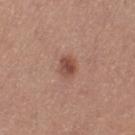follow-up: catalogued during a skin exam; not biopsied | location: the left thigh | illumination: white-light | size: about 2.5 mm | subject: female, roughly 40 years of age | image source: total-body-photography crop, ~15 mm field of view.